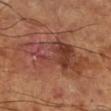<case>
<site>right lower leg</site>
<image>
  <source>total-body photography crop</source>
  <field_of_view_mm>15</field_of_view_mm>
</image>
<lesion_size>
  <long_diameter_mm_approx>10.0</long_diameter_mm_approx>
</lesion_size>
<patient>
  <sex>male</sex>
  <age_approx>65</age_approx>
</patient>
<lighting>cross-polarized</lighting>
<automated_metrics>
  <area_mm2_approx>33.0</area_mm2_approx>
  <eccentricity>0.85</eccentricity>
  <shape_asymmetry>0.35</shape_asymmetry>
  <cielab_L>42</cielab_L>
  <cielab_a>25</cielab_a>
  <cielab_b>28</cielab_b>
  <vs_skin_darker_L>9.0</vs_skin_darker_L>
  <vs_skin_contrast_norm>7.5</vs_skin_contrast_norm>
  <nevus_likeness_0_100>0</nevus_likeness_0_100>
  <lesion_detection_confidence_0_100>100</lesion_detection_confidence_0_100>
</automated_metrics>
</case>